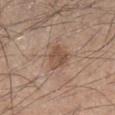Q: How was this image acquired?
A: ~15 mm crop, total-body skin-cancer survey
Q: What are the patient's age and sex?
A: male, about 45 years old
Q: Where on the body is the lesion?
A: the right lower leg